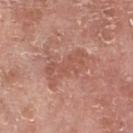Part of a total-body skin-imaging series; this lesion was reviewed on a skin check and was not flagged for biopsy. A male subject, aged around 75. From the right lower leg. A close-up tile cropped from a whole-body skin photograph, about 15 mm across.